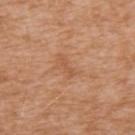Clinical summary:
Located on the upper back. A male subject approximately 75 years of age. The lesion-visualizer software estimated a footprint of about 2 mm², an outline eccentricity of about 0.95 (0 = round, 1 = elongated), and a symmetry-axis asymmetry near 0.35. And it measured internal color variation of about 0 on a 0–10 scale and peripheral color asymmetry of about 0. The tile uses white-light illumination. Measured at roughly 2.5 mm in maximum diameter. A lesion tile, about 15 mm wide, cut from a 3D total-body photograph.